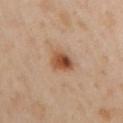Q: Was a biopsy performed?
A: catalogued during a skin exam; not biopsied